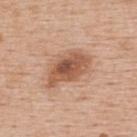Acquisition and patient details: A female patient roughly 65 years of age. Cropped from a whole-body photographic skin survey; the tile spans about 15 mm. Located on the upper back. Longest diameter approximately 6 mm. Captured under white-light illumination.